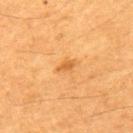Case summary:
- follow-up — total-body-photography surveillance lesion; no biopsy
- anatomic site — the back
- illumination — cross-polarized
- TBP lesion metrics — a lesion area of about 2.5 mm²; a mean CIELAB color near L≈57 a*≈25 b*≈47, about 9 CIELAB-L* units darker than the surrounding skin, and a lesion-to-skin contrast of about 6.5 (normalized; higher = more distinct); a lesion-detection confidence of about 100/100
- subject — male, aged 58 to 62
- image — 15 mm crop, total-body photography
- lesion size — ≈2.5 mm